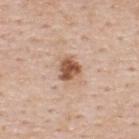Impression:
Part of a total-body skin-imaging series; this lesion was reviewed on a skin check and was not flagged for biopsy.
Background:
About 2.5 mm across. Automated image analysis of the tile measured a footprint of about 5.5 mm², an outline eccentricity of about 0.45 (0 = round, 1 = elongated), and two-axis asymmetry of about 0.25. It also reported a lesion color around L≈54 a*≈21 b*≈32 in CIELAB, roughly 16 lightness units darker than nearby skin, and a normalized lesion–skin contrast near 10.5. And it measured a classifier nevus-likeness of about 90/100 and a detector confidence of about 100 out of 100 that the crop contains a lesion. Captured under white-light illumination. A male subject, aged 48 to 52. Located on the upper back. Cropped from a whole-body photographic skin survey; the tile spans about 15 mm.